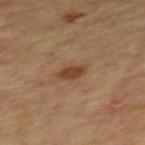biopsy_status: not biopsied; imaged during a skin examination